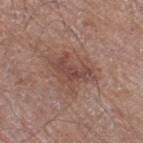diameter — ≈5.5 mm | patient — male, about 70 years old | image source — total-body-photography crop, ~15 mm field of view | body site — the right thigh | automated lesion analysis — a lesion color around L≈46 a*≈20 b*≈24 in CIELAB, roughly 9 lightness units darker than nearby skin, and a lesion-to-skin contrast of about 6.5 (normalized; higher = more distinct); internal color variation of about 3 on a 0–10 scale and peripheral color asymmetry of about 1; a nevus-likeness score of about 15/100 and a detector confidence of about 100 out of 100 that the crop contains a lesion | lighting — white-light.